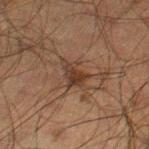This lesion was catalogued during total-body skin photography and was not selected for biopsy.
A male patient aged 48–52.
Approximately 3.5 mm at its widest.
On the right thigh.
A close-up tile cropped from a whole-body skin photograph, about 15 mm across.